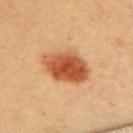This lesion was catalogued during total-body skin photography and was not selected for biopsy. The patient is a female about 60 years old. The lesion is located on the upper back. A lesion tile, about 15 mm wide, cut from a 3D total-body photograph.